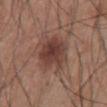Findings:
- follow-up: no biopsy performed (imaged during a skin exam)
- site: the abdomen
- automated metrics: a symmetry-axis asymmetry near 0.2; a lesion color around L≈40 a*≈20 b*≈24 in CIELAB, about 10 CIELAB-L* units darker than the surrounding skin, and a lesion-to-skin contrast of about 8.5 (normalized; higher = more distinct); a border-irregularity rating of about 2.5/10, internal color variation of about 5 on a 0–10 scale, and peripheral color asymmetry of about 1.5
- acquisition: ~15 mm crop, total-body skin-cancer survey
- subject: male, about 55 years old
- lighting: white-light